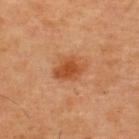Notes:
* biopsy status — no biopsy performed (imaged during a skin exam)
* anatomic site — the upper back
* image — total-body-photography crop, ~15 mm field of view
* patient — male, aged 43–47
* image-analysis metrics — an outline eccentricity of about 0.65 (0 = round, 1 = elongated) and two-axis asymmetry of about 0.3; a nevus-likeness score of about 95/100 and a detector confidence of about 100 out of 100 that the crop contains a lesion